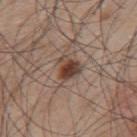Captured during whole-body skin photography for melanoma surveillance; the lesion was not biopsied.